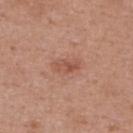<record>
<biopsy_status>not biopsied; imaged during a skin examination</biopsy_status>
<lesion_size>
  <long_diameter_mm_approx>3.0</long_diameter_mm_approx>
</lesion_size>
<image>
  <source>total-body photography crop</source>
  <field_of_view_mm>15</field_of_view_mm>
</image>
<lighting>white-light</lighting>
<site>upper back</site>
<automated_metrics>
  <area_mm2_approx>4.0</area_mm2_approx>
  <eccentricity>0.85</eccentricity>
</automated_metrics>
<patient>
  <sex>female</sex>
  <age_approx>40</age_approx>
</patient>
</record>